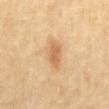The lesion was photographed on a routine skin check and not biopsied; there is no pathology result. A roughly 15 mm field-of-view crop from a total-body skin photograph. The lesion-visualizer software estimated about 10 CIELAB-L* units darker than the surrounding skin. The analysis additionally found lesion-presence confidence of about 100/100. On the mid back. The subject is a male aged 68 to 72.